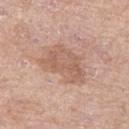Acquisition and patient details: The recorded lesion diameter is about 6 mm. A close-up tile cropped from a whole-body skin photograph, about 15 mm across. The lesion is on the left thigh. A female subject, aged 63 to 67.